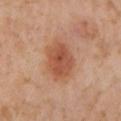follow-up: imaged on a skin check; not biopsied | lighting: cross-polarized illumination | body site: the left lower leg | subject: female, aged around 55 | image: ~15 mm crop, total-body skin-cancer survey | automated lesion analysis: a normalized lesion–skin contrast near 7.5; a border-irregularity rating of about 2/10 and a peripheral color-asymmetry measure near 1.5.